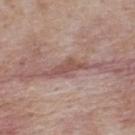Notes:
- follow-up — total-body-photography surveillance lesion; no biopsy
- location — the upper back
- subject — male, roughly 75 years of age
- imaging modality — ~15 mm crop, total-body skin-cancer survey
- illumination — white-light